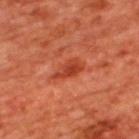biopsy status = total-body-photography surveillance lesion; no biopsy | site = the upper back | subject = male, aged around 65 | illumination = cross-polarized illumination | size = ≈4 mm | acquisition = ~15 mm tile from a whole-body skin photo.